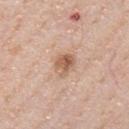Part of a total-body skin-imaging series; this lesion was reviewed on a skin check and was not flagged for biopsy. A male patient, about 40 years old. The recorded lesion diameter is about 3 mm. Cropped from a whole-body photographic skin survey; the tile spans about 15 mm. Imaged with white-light lighting. From the chest.